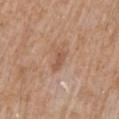Part of a total-body skin-imaging series; this lesion was reviewed on a skin check and was not flagged for biopsy.
Cropped from a total-body skin-imaging series; the visible field is about 15 mm.
The tile uses white-light illumination.
From the mid back.
The subject is a male aged around 60.
Longest diameter approximately 3 mm.
An algorithmic analysis of the crop reported a lesion color around L≈54 a*≈21 b*≈31 in CIELAB, roughly 8 lightness units darker than nearby skin, and a normalized lesion–skin contrast near 5.5. The analysis additionally found internal color variation of about 1 on a 0–10 scale and radial color variation of about 0.5.